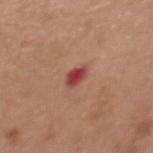Part of a total-body skin-imaging series; this lesion was reviewed on a skin check and was not flagged for biopsy. Longest diameter approximately 2.5 mm. A female patient aged approximately 50. A region of skin cropped from a whole-body photographic capture, roughly 15 mm wide. The lesion-visualizer software estimated an area of roughly 3.5 mm², an eccentricity of roughly 0.7, and two-axis asymmetry of about 0.25. The analysis additionally found a mean CIELAB color near L≈45 a*≈33 b*≈26, a lesion–skin lightness drop of about 13, and a lesion-to-skin contrast of about 10 (normalized; higher = more distinct). It also reported a border-irregularity index near 2/10, a within-lesion color-variation index near 2/10, and radial color variation of about 0.5. The analysis additionally found a classifier nevus-likeness of about 0/100 and lesion-presence confidence of about 100/100. The lesion is on the upper back. The tile uses white-light illumination.